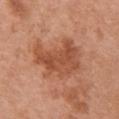Captured during whole-body skin photography for melanoma surveillance; the lesion was not biopsied. The total-body-photography lesion software estimated a border-irregularity rating of about 5.5/10 and a color-variation rating of about 3.5/10. It also reported an automated nevus-likeness rating near 45 out of 100 and a detector confidence of about 100 out of 100 that the crop contains a lesion. The tile uses white-light illumination. The subject is a female aged 63–67. Located on the arm. The recorded lesion diameter is about 6 mm. Cropped from a whole-body photographic skin survey; the tile spans about 15 mm.